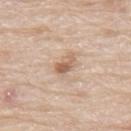| key | value |
|---|---|
| workup | catalogued during a skin exam; not biopsied |
| image source | total-body-photography crop, ~15 mm field of view |
| patient | male, approximately 80 years of age |
| location | the mid back |